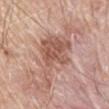notes: catalogued during a skin exam; not biopsied
image source: total-body-photography crop, ~15 mm field of view
subject: male, about 80 years old
anatomic site: the mid back